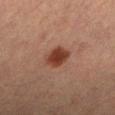Part of a total-body skin-imaging series; this lesion was reviewed on a skin check and was not flagged for biopsy. The subject is a female roughly 60 years of age. Automated image analysis of the tile measured a lesion area of about 7 mm², an eccentricity of roughly 0.45, and a symmetry-axis asymmetry near 0.15. It also reported about 11 CIELAB-L* units darker than the surrounding skin and a normalized lesion–skin contrast near 10. The software also gave a border-irregularity rating of about 1.5/10, a within-lesion color-variation index near 2/10, and peripheral color asymmetry of about 0.5. It also reported a nevus-likeness score of about 100/100 and a detector confidence of about 100 out of 100 that the crop contains a lesion. The lesion is on the left lower leg. A lesion tile, about 15 mm wide, cut from a 3D total-body photograph.